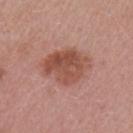biopsy_status: not biopsied; imaged during a skin examination
lesion_size:
  long_diameter_mm_approx: 6.0
patient:
  sex: female
  age_approx: 45
image:
  source: total-body photography crop
  field_of_view_mm: 15
site: left upper arm